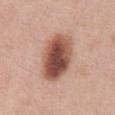{"biopsy_status": "not biopsied; imaged during a skin examination", "site": "abdomen", "lesion_size": {"long_diameter_mm_approx": 6.0}, "image": {"source": "total-body photography crop", "field_of_view_mm": 15}, "lighting": "white-light", "automated_metrics": {"area_mm2_approx": 19.0, "shape_asymmetry": 0.15}, "patient": {"sex": "male", "age_approx": 60}}